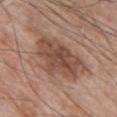biopsy status = imaged on a skin check; not biopsied
acquisition = 15 mm crop, total-body photography
location = the chest
patient = male, approximately 70 years of age
tile lighting = white-light illumination